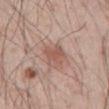The lesion was tiled from a total-body skin photograph and was not biopsied. From the abdomen. A male patient, aged 53 to 57. A 15 mm close-up extracted from a 3D total-body photography capture.